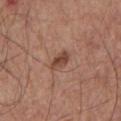biopsy_status: not biopsied; imaged during a skin examination